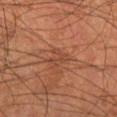site: the right lower leg
acquisition: 15 mm crop, total-body photography
TBP lesion metrics: an outline eccentricity of about 0.6 (0 = round, 1 = elongated) and a symmetry-axis asymmetry near 0.5; border irregularity of about 7.5 on a 0–10 scale
diameter: about 2.5 mm
patient: male, in their mid- to late 50s
illumination: cross-polarized